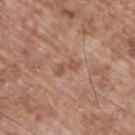workup: imaged on a skin check; not biopsied
patient: male, aged around 65
tile lighting: white-light illumination
anatomic site: the upper back
acquisition: 15 mm crop, total-body photography
size: ~3 mm (longest diameter)